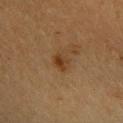  biopsy_status: not biopsied; imaged during a skin examination
  lighting: cross-polarized
  site: right forearm
  patient:
    sex: female
    age_approx: 40
  image:
    source: total-body photography crop
    field_of_view_mm: 15